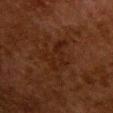biopsy_status: not biopsied; imaged during a skin examination
site: upper back
image:
  source: total-body photography crop
  field_of_view_mm: 15
lighting: cross-polarized
lesion_size:
  long_diameter_mm_approx: 4.5
patient:
  sex: female
  age_approx: 50